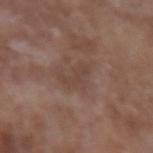<lesion>
<biopsy_status>not biopsied; imaged during a skin examination</biopsy_status>
<automated_metrics>
  <eccentricity>0.85</eccentricity>
  <cielab_L>42</cielab_L>
  <cielab_a>17</cielab_a>
  <cielab_b>23</cielab_b>
  <vs_skin_darker_L>6.0</vs_skin_darker_L>
  <vs_skin_contrast_norm>5.0</vs_skin_contrast_norm>
  <lesion_detection_confidence_0_100>100</lesion_detection_confidence_0_100>
</automated_metrics>
<lesion_size>
  <long_diameter_mm_approx>3.5</long_diameter_mm_approx>
</lesion_size>
<patient>
  <sex>male</sex>
  <age_approx>65</age_approx>
</patient>
<lighting>white-light</lighting>
<site>left upper arm</site>
<image>
  <source>total-body photography crop</source>
  <field_of_view_mm>15</field_of_view_mm>
</image>
</lesion>